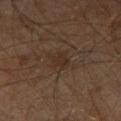{"biopsy_status": "not biopsied; imaged during a skin examination", "lesion_size": {"long_diameter_mm_approx": 2.5}, "patient": {"sex": "male", "age_approx": 60}, "site": "right leg", "lighting": "cross-polarized", "image": {"source": "total-body photography crop", "field_of_view_mm": 15}, "automated_metrics": {"shape_asymmetry": 0.25, "cielab_L": 27, "cielab_a": 14, "cielab_b": 23, "vs_skin_contrast_norm": 5.5, "border_irregularity_0_10": 3.0, "color_variation_0_10": 1.5, "nevus_likeness_0_100": 0, "lesion_detection_confidence_0_100": 100}}